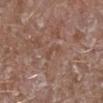Q: Is there a histopathology result?
A: no biopsy performed (imaged during a skin exam)
Q: Patient demographics?
A: male, aged 43 to 47
Q: How was this image acquired?
A: total-body-photography crop, ~15 mm field of view
Q: How was the tile lit?
A: white-light
Q: Automated lesion metrics?
A: a footprint of about 2.5 mm² and a shape-asymmetry score of about 0.35 (0 = symmetric); a lesion color around L≈47 a*≈19 b*≈26 in CIELAB and a normalized lesion–skin contrast near 4.5; a nevus-likeness score of about 0/100
Q: Where on the body is the lesion?
A: the right lower leg
Q: Lesion size?
A: ~2.5 mm (longest diameter)